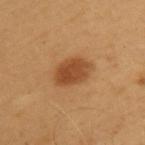  biopsy_status: not biopsied; imaged during a skin examination
  site: back
  image:
    source: total-body photography crop
    field_of_view_mm: 15
  patient:
    sex: male
    age_approx: 55
  lesion_size:
    long_diameter_mm_approx: 4.5